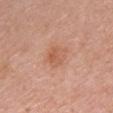Assessment:
Part of a total-body skin-imaging series; this lesion was reviewed on a skin check and was not flagged for biopsy.
Clinical summary:
Automated tile analysis of the lesion measured an area of roughly 6 mm² and two-axis asymmetry of about 0.2. And it measured about 7 CIELAB-L* units darker than the surrounding skin and a normalized border contrast of about 5.5. The software also gave a border-irregularity rating of about 2/10 and a color-variation rating of about 4/10. On the chest. This image is a 15 mm lesion crop taken from a total-body photograph. A female patient, aged around 50. Measured at roughly 3 mm in maximum diameter.